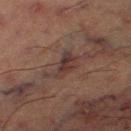Part of a total-body skin-imaging series; this lesion was reviewed on a skin check and was not flagged for biopsy.
A 15 mm close-up extracted from a 3D total-body photography capture.
Located on the left thigh.
Approximately 4.5 mm at its widest.
A male subject, aged around 65.